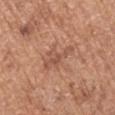{
  "biopsy_status": "not biopsied; imaged during a skin examination",
  "automated_metrics": {
    "area_mm2_approx": 6.5,
    "shape_asymmetry": 0.4
  },
  "patient": {
    "sex": "male",
    "age_approx": 75
  },
  "lesion_size": {
    "long_diameter_mm_approx": 4.5
  },
  "site": "arm",
  "image": {
    "source": "total-body photography crop",
    "field_of_view_mm": 15
  }
}